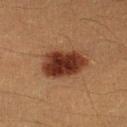{
  "biopsy_status": "not biopsied; imaged during a skin examination",
  "patient": {
    "sex": "male",
    "age_approx": 40
  },
  "lighting": "cross-polarized",
  "image": {
    "source": "total-body photography crop",
    "field_of_view_mm": 15
  },
  "site": "left lower leg",
  "lesion_size": {
    "long_diameter_mm_approx": 5.5
  }
}